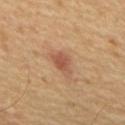Context:
Located on the upper back. Captured under cross-polarized illumination. The subject is a male aged around 65. Approximately 3.5 mm at its widest. Cropped from a whole-body photographic skin survey; the tile spans about 15 mm.